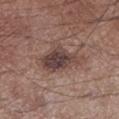| feature | finding |
|---|---|
| biopsy status | catalogued during a skin exam; not biopsied |
| image source | 15 mm crop, total-body photography |
| location | the left lower leg |
| subject | male, about 55 years old |